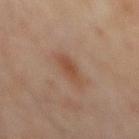<case>
  <biopsy_status>not biopsied; imaged during a skin examination</biopsy_status>
  <patient>
    <sex>male</sex>
    <age_approx>65</age_approx>
  </patient>
  <lesion_size>
    <long_diameter_mm_approx>4.0</long_diameter_mm_approx>
  </lesion_size>
  <site>mid back</site>
  <image>
    <source>total-body photography crop</source>
    <field_of_view_mm>15</field_of_view_mm>
  </image>
  <automated_metrics>
    <area_mm2_approx>6.0</area_mm2_approx>
    <eccentricity>0.9</eccentricity>
    <border_irregularity_0_10>2.5</border_irregularity_0_10>
    <color_variation_0_10>2.5</color_variation_0_10>
  </automated_metrics>
</case>